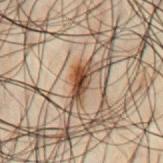The lesion was photographed on a routine skin check and not biopsied; there is no pathology result. A male subject, aged around 50. A 15 mm close-up tile from a total-body photography series done for melanoma screening. The lesion is on the chest. Imaged with cross-polarized lighting. Automated tile analysis of the lesion measured an outline eccentricity of about 0.9 (0 = round, 1 = elongated) and a shape-asymmetry score of about 0.3 (0 = symmetric). The software also gave an average lesion color of about L≈33 a*≈15 b*≈25 (CIELAB) and a normalized lesion–skin contrast near 12.5. It also reported a border-irregularity rating of about 3.5/10, a within-lesion color-variation index near 6/10, and peripheral color asymmetry of about 2.5. The software also gave a classifier nevus-likeness of about 100/100 and lesion-presence confidence of about 100/100. Approximately 3.5 mm at its widest.